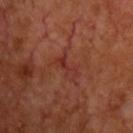Recorded during total-body skin imaging; not selected for excision or biopsy.
On the chest.
Cropped from a total-body skin-imaging series; the visible field is about 15 mm.
A male patient in their mid-60s.